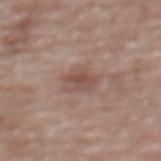Recorded during total-body skin imaging; not selected for excision or biopsy. A roughly 15 mm field-of-view crop from a total-body skin photograph. Imaged with white-light lighting. A female patient, roughly 65 years of age. The lesion-visualizer software estimated a border-irregularity index near 3/10 and a color-variation rating of about 4/10. The software also gave an automated nevus-likeness rating near 0 out of 100 and lesion-presence confidence of about 100/100. Approximately 4 mm at its widest. The lesion is on the back.